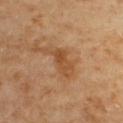{"lesion_size": {"long_diameter_mm_approx": 5.0}, "lighting": "cross-polarized", "patient": {"sex": "male", "age_approx": "85+"}, "site": "upper back", "image": {"source": "total-body photography crop", "field_of_view_mm": 15}}